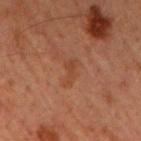Captured during whole-body skin photography for melanoma surveillance; the lesion was not biopsied.
A region of skin cropped from a whole-body photographic capture, roughly 15 mm wide.
The subject is a male aged 58 to 62.
The recorded lesion diameter is about 2.5 mm.
The tile uses cross-polarized illumination.
The lesion is located on the chest.